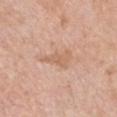This lesion was catalogued during total-body skin photography and was not selected for biopsy.
Automated image analysis of the tile measured a lesion area of about 6 mm², a shape eccentricity near 0.85, and two-axis asymmetry of about 0.5. The analysis additionally found a mean CIELAB color near L≈63 a*≈20 b*≈33, about 7 CIELAB-L* units darker than the surrounding skin, and a lesion-to-skin contrast of about 5.5 (normalized; higher = more distinct).
A female patient aged 58–62.
The lesion's longest dimension is about 4 mm.
On the front of the torso.
Captured under white-light illumination.
A 15 mm close-up tile from a total-body photography series done for melanoma screening.